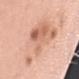Assessment:
This lesion was catalogued during total-body skin photography and was not selected for biopsy.
Image and clinical context:
From the left upper arm. Automated image analysis of the tile measured an area of roughly 33 mm² and a symmetry-axis asymmetry near 0.35. And it measured a border-irregularity index near 4/10, internal color variation of about 8.5 on a 0–10 scale, and radial color variation of about 3. It also reported lesion-presence confidence of about 100/100. A female subject, approximately 50 years of age. Cropped from a total-body skin-imaging series; the visible field is about 15 mm.